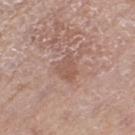{"biopsy_status": "not biopsied; imaged during a skin examination", "lesion_size": {"long_diameter_mm_approx": 3.5}, "patient": {"sex": "female", "age_approx": 75}, "image": {"source": "total-body photography crop", "field_of_view_mm": 15}, "site": "left thigh", "lighting": "white-light"}